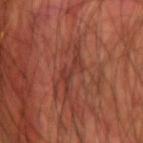notes — total-body-photography surveillance lesion; no biopsy | tile lighting — cross-polarized | body site — the left upper arm | lesion size — ~5.5 mm (longest diameter) | image — total-body-photography crop, ~15 mm field of view | patient — male, roughly 60 years of age | automated metrics — a footprint of about 6.5 mm², an outline eccentricity of about 0.95 (0 = round, 1 = elongated), and a shape-asymmetry score of about 0.65 (0 = symmetric); a mean CIELAB color near L≈37 a*≈27 b*≈28, roughly 7 lightness units darker than nearby skin, and a lesion-to-skin contrast of about 6 (normalized; higher = more distinct); a nevus-likeness score of about 5/100 and a lesion-detection confidence of about 80/100.